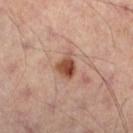Impression:
The lesion was tiled from a total-body skin photograph and was not biopsied.
Context:
A 15 mm crop from a total-body photograph taken for skin-cancer surveillance. On the left leg. A male patient aged approximately 50.